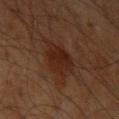{"site": "right upper arm", "image": {"source": "total-body photography crop", "field_of_view_mm": 15}, "lighting": "cross-polarized", "patient": {"sex": "male", "age_approx": 60}, "lesion_size": {"long_diameter_mm_approx": 3.5}}